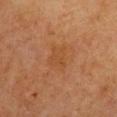Impression: Imaged during a routine full-body skin examination; the lesion was not biopsied and no histopathology is available. Acquisition and patient details: The tile uses cross-polarized illumination. A female patient in their mid- to late 50s. On the chest. A 15 mm close-up tile from a total-body photography series done for melanoma screening. The lesion-visualizer software estimated a mean CIELAB color near L≈40 a*≈20 b*≈33, a lesion–skin lightness drop of about 4, and a lesion-to-skin contrast of about 4.5 (normalized; higher = more distinct). It also reported border irregularity of about 2.5 on a 0–10 scale and a within-lesion color-variation index near 2/10. The software also gave a lesion-detection confidence of about 100/100. Longest diameter approximately 3.5 mm.